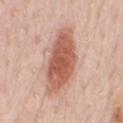Recorded during total-body skin imaging; not selected for excision or biopsy.
An algorithmic analysis of the crop reported an outline eccentricity of about 0.9 (0 = round, 1 = elongated) and a symmetry-axis asymmetry near 0.2.
The lesion is located on the front of the torso.
A male patient about 60 years old.
A close-up tile cropped from a whole-body skin photograph, about 15 mm across.
The tile uses white-light illumination.